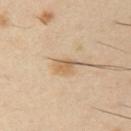notes = catalogued during a skin exam; not biopsied
site = the right upper arm
subject = male, aged 38–42
lesion diameter = ~2.5 mm (longest diameter)
tile lighting = cross-polarized illumination
image = 15 mm crop, total-body photography